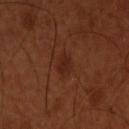The lesion was tiled from a total-body skin photograph and was not biopsied.
About 3 mm across.
A region of skin cropped from a whole-body photographic capture, roughly 15 mm wide.
The lesion is located on the head or neck.
A male subject aged 53 to 57.
Imaged with cross-polarized lighting.